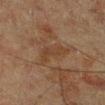Captured during whole-body skin photography for melanoma surveillance; the lesion was not biopsied.
A 15 mm close-up extracted from a 3D total-body photography capture.
Captured under cross-polarized illumination.
A male patient about 60 years old.
The lesion is located on the left lower leg.